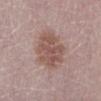* workup — total-body-photography surveillance lesion; no biopsy
* automated lesion analysis — peripheral color asymmetry of about 1.5
* subject — female, aged approximately 50
* body site — the leg
* image source — ~15 mm tile from a whole-body skin photo